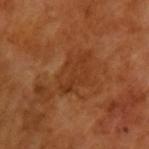biopsy_status: not biopsied; imaged during a skin examination
image:
  source: total-body photography crop
  field_of_view_mm: 15
lighting: cross-polarized
patient:
  sex: male
  age_approx: 65
lesion_size:
  long_diameter_mm_approx: 5.0